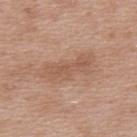follow-up: catalogued during a skin exam; not biopsied | subject: female, approximately 40 years of age | imaging modality: total-body-photography crop, ~15 mm field of view | diameter: about 6.5 mm | site: the upper back.